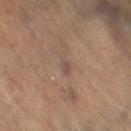follow-up: imaged on a skin check; not biopsied | illumination: cross-polarized | anatomic site: the right lower leg | lesion size: ≈2.5 mm | acquisition: ~15 mm tile from a whole-body skin photo | automated lesion analysis: a lesion color around L≈48 a*≈15 b*≈22 in CIELAB and a normalized lesion–skin contrast near 6; a border-irregularity index near 2/10, a color-variation rating of about 0.5/10, and radial color variation of about 0 | patient: female, in their mid-60s.